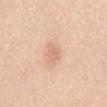<lesion>
  <patient>
    <sex>female</sex>
    <age_approx>70</age_approx>
  </patient>
  <lighting>white-light</lighting>
  <image>
    <source>total-body photography crop</source>
    <field_of_view_mm>15</field_of_view_mm>
  </image>
  <automated_metrics>
    <area_mm2_approx>4.0</area_mm2_approx>
    <eccentricity>0.8</eccentricity>
    <shape_asymmetry>0.35</shape_asymmetry>
    <cielab_L>70</cielab_L>
    <cielab_a>20</cielab_a>
    <cielab_b>33</cielab_b>
    <vs_skin_darker_L>8.0</vs_skin_darker_L>
    <vs_skin_contrast_norm>5.0</vs_skin_contrast_norm>
    <border_irregularity_0_10>3.5</border_irregularity_0_10>
    <color_variation_0_10>1.5</color_variation_0_10>
    <peripheral_color_asymmetry>0.5</peripheral_color_asymmetry>
  </automated_metrics>
  <site>mid back</site>
  <lesion_size>
    <long_diameter_mm_approx>3.0</long_diameter_mm_approx>
  </lesion_size>
</lesion>